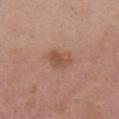| feature | finding |
|---|---|
| follow-up | total-body-photography surveillance lesion; no biopsy |